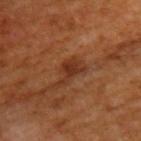biopsy status = total-body-photography surveillance lesion; no biopsy | image = ~15 mm crop, total-body skin-cancer survey | subject = male, aged around 65 | body site = the upper back.